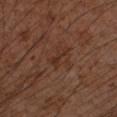Captured during whole-body skin photography for melanoma surveillance; the lesion was not biopsied. The lesion is located on the left forearm. Cropped from a total-body skin-imaging series; the visible field is about 15 mm. The recorded lesion diameter is about 2.5 mm. A male patient in their mid-50s.